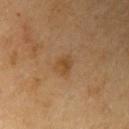Assessment: This lesion was catalogued during total-body skin photography and was not selected for biopsy. Background: Cropped from a total-body skin-imaging series; the visible field is about 15 mm. On the right upper arm. A male subject, roughly 70 years of age.